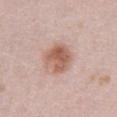Q: Was a biopsy performed?
A: no biopsy performed (imaged during a skin exam)
Q: How was this image acquired?
A: total-body-photography crop, ~15 mm field of view
Q: Who is the patient?
A: female, about 40 years old
Q: What is the lesion's diameter?
A: about 4 mm
Q: What is the anatomic site?
A: the abdomen
Q: Illumination type?
A: white-light